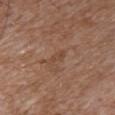Q: Was this lesion biopsied?
A: total-body-photography surveillance lesion; no biopsy
Q: How was this image acquired?
A: ~15 mm crop, total-body skin-cancer survey
Q: Patient demographics?
A: female, about 85 years old or older
Q: What is the anatomic site?
A: the chest